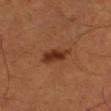follow-up = imaged on a skin check; not biopsied | automated metrics = a lesion area of about 6.5 mm²; an average lesion color of about L≈34 a*≈24 b*≈31 (CIELAB) | body site = the right thigh | imaging modality = ~15 mm tile from a whole-body skin photo | lighting = cross-polarized illumination | patient = male, aged 48 to 52.